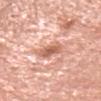Impression:
The lesion was photographed on a routine skin check and not biopsied; there is no pathology result.
Acquisition and patient details:
The tile uses white-light illumination. Automated image analysis of the tile measured a footprint of about 7 mm², an outline eccentricity of about 0.8 (0 = round, 1 = elongated), and a symmetry-axis asymmetry near 0.25. The analysis additionally found a lesion color around L≈64 a*≈25 b*≈31 in CIELAB, a lesion–skin lightness drop of about 12, and a normalized lesion–skin contrast near 7.5. The analysis additionally found a classifier nevus-likeness of about 0/100 and a lesion-detection confidence of about 95/100. A female subject about 65 years old. About 4 mm across. This image is a 15 mm lesion crop taken from a total-body photograph. Located on the head or neck.